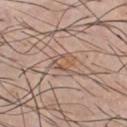follow-up: imaged on a skin check; not biopsied | diameter: ~3 mm (longest diameter) | image-analysis metrics: an area of roughly 5.5 mm², a shape eccentricity near 0.55, and a symmetry-axis asymmetry near 0.4 | acquisition: ~15 mm crop, total-body skin-cancer survey | illumination: white-light | location: the chest | subject: male, aged 43–47.